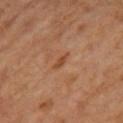The lesion was tiled from a total-body skin photograph and was not biopsied.
From the right thigh.
A male patient, roughly 55 years of age.
Longest diameter approximately 2.5 mm.
Cropped from a total-body skin-imaging series; the visible field is about 15 mm.
Imaged with cross-polarized lighting.